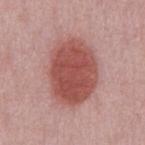<case>
  <image>
    <source>total-body photography crop</source>
    <field_of_view_mm>15</field_of_view_mm>
  </image>
  <site>chest</site>
  <lighting>white-light</lighting>
  <patient>
    <sex>male</sex>
    <age_approx>50</age_approx>
  </patient>
</case>